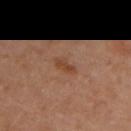follow-up: no biopsy performed (imaged during a skin exam)
automated lesion analysis: an area of roughly 3 mm², an outline eccentricity of about 0.9 (0 = round, 1 = elongated), and two-axis asymmetry of about 0.3; an average lesion color of about L≈41 a*≈21 b*≈31 (CIELAB) and a lesion–skin lightness drop of about 7; a color-variation rating of about 0/10 and a peripheral color-asymmetry measure near 0
site: the left upper arm
patient: female, aged 48 to 52
lesion size: about 3 mm
acquisition: total-body-photography crop, ~15 mm field of view